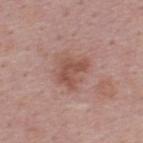Clinical impression: The lesion was photographed on a routine skin check and not biopsied; there is no pathology result. Context: Approximately 3.5 mm at its widest. A male patient aged 48 to 52. This is a white-light tile. The total-body-photography lesion software estimated a lesion color around L≈51 a*≈22 b*≈26 in CIELAB, a lesion–skin lightness drop of about 9, and a normalized border contrast of about 7. And it measured border irregularity of about 4.5 on a 0–10 scale and a color-variation rating of about 2/10. From the upper back. A region of skin cropped from a whole-body photographic capture, roughly 15 mm wide.